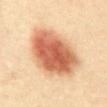follow-up: no biopsy performed (imaged during a skin exam)
body site: the mid back
patient: female, about 40 years old
image source: ~15 mm crop, total-body skin-cancer survey
automated lesion analysis: border irregularity of about 2 on a 0–10 scale, internal color variation of about 8.5 on a 0–10 scale, and peripheral color asymmetry of about 3